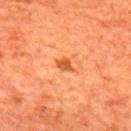  automated_metrics:
    eccentricity: 0.75
    shape_asymmetry: 0.25
    border_irregularity_0_10: 2.5
    color_variation_0_10: 1.5
    peripheral_color_asymmetry: 0.5
    nevus_likeness_0_100: 85
  image:
    source: total-body photography crop
    field_of_view_mm: 15
  lighting: cross-polarized
  patient:
    sex: male
    age_approx: 65
  site: upper back
  lesion_size:
    long_diameter_mm_approx: 2.5